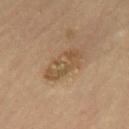An algorithmic analysis of the crop reported an average lesion color of about L≈50 a*≈15 b*≈33 (CIELAB), roughly 8 lightness units darker than nearby skin, and a normalized lesion–skin contrast near 6. And it measured a classifier nevus-likeness of about 0/100 and a lesion-detection confidence of about 100/100. Cropped from a whole-body photographic skin survey; the tile spans about 15 mm. The recorded lesion diameter is about 5 mm. On the mid back. The tile uses cross-polarized illumination. The patient is a male aged 83 or over.